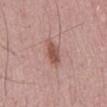Findings:
* notes · total-body-photography surveillance lesion; no biopsy
* lesion size · about 4.5 mm
* patient · male, aged 48 to 52
* imaging modality · 15 mm crop, total-body photography
* illumination · white-light
* site · the mid back